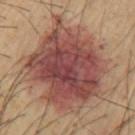Notes:
* notes · no biopsy performed (imaged during a skin exam)
* tile lighting · cross-polarized
* automated lesion analysis · a footprint of about 65 mm², an outline eccentricity of about 0.6 (0 = round, 1 = elongated), and two-axis asymmetry of about 0.2; an automated nevus-likeness rating near 80 out of 100 and a lesion-detection confidence of about 100/100
* subject · male, approximately 60 years of age
* body site · the left upper arm
* imaging modality · ~15 mm crop, total-body skin-cancer survey
* diameter · ~10 mm (longest diameter)